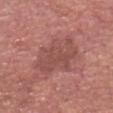This lesion was catalogued during total-body skin photography and was not selected for biopsy.
From the head or neck.
The tile uses white-light illumination.
A 15 mm crop from a total-body photograph taken for skin-cancer surveillance.
A male patient, aged 78–82.
The lesion's longest dimension is about 5.5 mm.